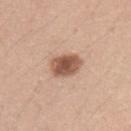<tbp_lesion>
<patient>
  <sex>female</sex>
  <age_approx>25</age_approx>
</patient>
<automated_metrics>
  <area_mm2_approx>8.5</area_mm2_approx>
  <eccentricity>0.8</eccentricity>
  <peripheral_color_asymmetry>1.0</peripheral_color_asymmetry>
  <lesion_detection_confidence_0_100>100</lesion_detection_confidence_0_100>
</automated_metrics>
<lesion_size>
  <long_diameter_mm_approx>4.0</long_diameter_mm_approx>
</lesion_size>
<lighting>white-light</lighting>
<image>
  <source>total-body photography crop</source>
  <field_of_view_mm>15</field_of_view_mm>
</image>
<site>arm</site>
</tbp_lesion>